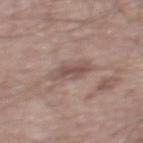<tbp_lesion>
<lighting>white-light</lighting>
<image>
  <source>total-body photography crop</source>
  <field_of_view_mm>15</field_of_view_mm>
</image>
<lesion_size>
  <long_diameter_mm_approx>4.0</long_diameter_mm_approx>
</lesion_size>
<site>mid back</site>
<patient>
  <sex>male</sex>
  <age_approx>55</age_approx>
</patient>
</tbp_lesion>